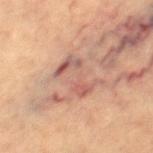Imaged during a routine full-body skin examination; the lesion was not biopsied and no histopathology is available. A lesion tile, about 15 mm wide, cut from a 3D total-body photograph. The recorded lesion diameter is about 4.5 mm. The patient is a female in their mid-60s. On the leg. The total-body-photography lesion software estimated a footprint of about 9 mm², an outline eccentricity of about 0.8 (0 = round, 1 = elongated), and a symmetry-axis asymmetry near 0.5. The software also gave a peripheral color-asymmetry measure near 3. And it measured an automated nevus-likeness rating near 0 out of 100 and a lesion-detection confidence of about 90/100.